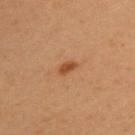biopsy status = total-body-photography surveillance lesion; no biopsy | patient = male, in their mid-30s | body site = the upper back | diameter = ≈2.5 mm | image = ~15 mm tile from a whole-body skin photo | illumination = cross-polarized.